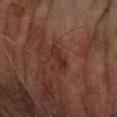Q: Lesion location?
A: the right forearm
Q: Patient demographics?
A: male, aged 73 to 77
Q: What is the imaging modality?
A: ~15 mm crop, total-body skin-cancer survey
Q: Lesion size?
A: about 3 mm